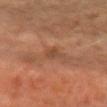Assessment:
Imaged during a routine full-body skin examination; the lesion was not biopsied and no histopathology is available.